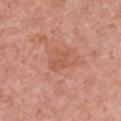The lesion is located on the chest. The total-body-photography lesion software estimated a normalized border contrast of about 4.5. A female patient about 60 years old. Imaged with white-light lighting. A region of skin cropped from a whole-body photographic capture, roughly 15 mm wide. Measured at roughly 3 mm in maximum diameter.